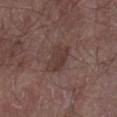Clinical summary:
A male subject, in their mid-60s. Captured under white-light illumination. From the arm. An algorithmic analysis of the crop reported a classifier nevus-likeness of about 0/100 and a detector confidence of about 100 out of 100 that the crop contains a lesion. Measured at roughly 3.5 mm in maximum diameter. Cropped from a whole-body photographic skin survey; the tile spans about 15 mm.